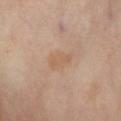The lesion was tiled from a total-body skin photograph and was not biopsied.
Located on the abdomen.
A female subject in their mid- to late 50s.
The recorded lesion diameter is about 3.5 mm.
Automated tile analysis of the lesion measured a classifier nevus-likeness of about 0/100 and a detector confidence of about 100 out of 100 that the crop contains a lesion.
A lesion tile, about 15 mm wide, cut from a 3D total-body photograph.
Imaged with cross-polarized lighting.